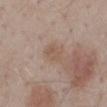workup = total-body-photography surveillance lesion; no biopsy | location = the mid back | imaging modality = ~15 mm crop, total-body skin-cancer survey | automated metrics = a footprint of about 4 mm², a shape eccentricity near 0.75, and a symmetry-axis asymmetry near 0.25; border irregularity of about 2.5 on a 0–10 scale and a peripheral color-asymmetry measure near 0.5; lesion-presence confidence of about 100/100 | subject = male, in their mid-50s | illumination = white-light illumination.